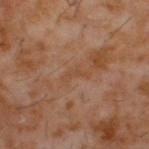– follow-up: total-body-photography surveillance lesion; no biopsy
– image: ~15 mm crop, total-body skin-cancer survey
– body site: the upper back
– size: ≈3 mm
– illumination: cross-polarized
– patient: male, in their 60s